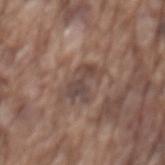workup: catalogued during a skin exam; not biopsied
diameter: about 4 mm
TBP lesion metrics: a lesion area of about 6.5 mm², a shape eccentricity near 0.85, and a shape-asymmetry score of about 0.4 (0 = symmetric); a lesion color around L≈44 a*≈16 b*≈21 in CIELAB and a normalized lesion–skin contrast near 7; border irregularity of about 6.5 on a 0–10 scale and internal color variation of about 3 on a 0–10 scale; an automated nevus-likeness rating near 0 out of 100 and a lesion-detection confidence of about 75/100
site: the mid back
patient: male, approximately 75 years of age
image source: 15 mm crop, total-body photography